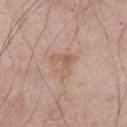Clinical impression: Recorded during total-body skin imaging; not selected for excision or biopsy. Clinical summary: The lesion is located on the leg. Measured at roughly 3.5 mm in maximum diameter. Imaged with white-light lighting. A roughly 15 mm field-of-view crop from a total-body skin photograph. A male patient about 55 years old.